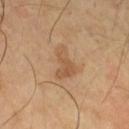Q: Was a biopsy performed?
A: imaged on a skin check; not biopsied
Q: What is the imaging modality?
A: ~15 mm tile from a whole-body skin photo
Q: Who is the patient?
A: male, about 65 years old
Q: How was the tile lit?
A: cross-polarized illumination
Q: What is the anatomic site?
A: the right thigh